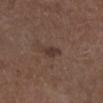Part of a total-body skin-imaging series; this lesion was reviewed on a skin check and was not flagged for biopsy.